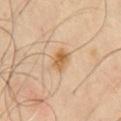Findings:
- notes: total-body-photography surveillance lesion; no biopsy
- body site: the chest
- patient: male, about 45 years old
- automated metrics: roughly 10 lightness units darker than nearby skin and a normalized border contrast of about 8; border irregularity of about 1.5 on a 0–10 scale and peripheral color asymmetry of about 1.5
- lesion diameter: ≈3 mm
- image: ~15 mm crop, total-body skin-cancer survey